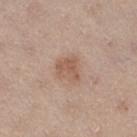| feature | finding |
|---|---|
| diameter | ~3 mm (longest diameter) |
| automated lesion analysis | a lesion area of about 5 mm²; a lesion color around L≈56 a*≈19 b*≈29 in CIELAB and a normalized lesion–skin contrast near 6.5; an automated nevus-likeness rating near 25 out of 100 and a lesion-detection confidence of about 100/100 |
| image | ~15 mm crop, total-body skin-cancer survey |
| illumination | white-light |
| body site | the right thigh |
| subject | female, in their 40s |